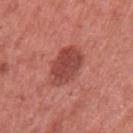Clinical impression:
Captured during whole-body skin photography for melanoma surveillance; the lesion was not biopsied.
Clinical summary:
The lesion's longest dimension is about 5 mm. Automated image analysis of the tile measured internal color variation of about 3.5 on a 0–10 scale and radial color variation of about 1. The patient is a female aged 48–52. The lesion is located on the left upper arm. A 15 mm crop from a total-body photograph taken for skin-cancer surveillance. Captured under white-light illumination.